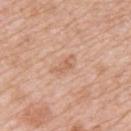Captured during whole-body skin photography for melanoma surveillance; the lesion was not biopsied.